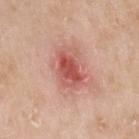Q: Is there a histopathology result?
A: imaged on a skin check; not biopsied
Q: Lesion location?
A: the arm
Q: What lighting was used for the tile?
A: white-light
Q: What kind of image is this?
A: 15 mm crop, total-body photography
Q: Lesion size?
A: ~4 mm (longest diameter)
Q: Patient demographics?
A: female, about 55 years old